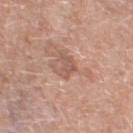Assessment:
The lesion was tiled from a total-body skin photograph and was not biopsied.
Clinical summary:
Imaged with white-light lighting. The lesion is located on the left thigh. Cropped from a total-body skin-imaging series; the visible field is about 15 mm. The subject is a female roughly 75 years of age.